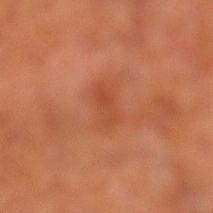  biopsy_status: not biopsied; imaged during a skin examination
  lighting: cross-polarized
  site: leg
  automated_metrics:
    lesion_detection_confidence_0_100: 100
  image:
    source: total-body photography crop
    field_of_view_mm: 15
  lesion_size:
    long_diameter_mm_approx: 3.5
  patient:
    sex: male
    age_approx: 70